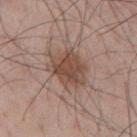Image and clinical context:
The patient is a male in their mid-50s. Located on the mid back. A close-up tile cropped from a whole-body skin photograph, about 15 mm across.